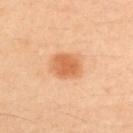Q: Was a biopsy performed?
A: no biopsy performed (imaged during a skin exam)
Q: Patient demographics?
A: male, aged 38 to 42
Q: How was this image acquired?
A: ~15 mm crop, total-body skin-cancer survey
Q: What lighting was used for the tile?
A: cross-polarized illumination
Q: Lesion location?
A: the left upper arm
Q: Automated lesion metrics?
A: roughly 11 lightness units darker than nearby skin and a normalized border contrast of about 7.5